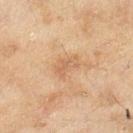Recorded during total-body skin imaging; not selected for excision or biopsy. Measured at roughly 3 mm in maximum diameter. Automated tile analysis of the lesion measured a mean CIELAB color near L≈57 a*≈19 b*≈33, a lesion–skin lightness drop of about 7, and a normalized lesion–skin contrast near 5. And it measured a classifier nevus-likeness of about 0/100. A female subject, roughly 60 years of age. Located on the right lower leg. This is a cross-polarized tile. A roughly 15 mm field-of-view crop from a total-body skin photograph.